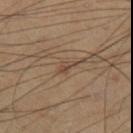No biopsy was performed on this lesion — it was imaged during a full skin examination and was not determined to be concerning.
Cropped from a whole-body photographic skin survey; the tile spans about 15 mm.
From the right thigh.
Automated image analysis of the tile measured an average lesion color of about L≈43 a*≈15 b*≈26 (CIELAB). And it measured a peripheral color-asymmetry measure near 0.5. It also reported a classifier nevus-likeness of about 5/100 and lesion-presence confidence of about 60/100.
The patient is a male aged around 70.